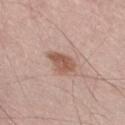Notes:
– follow-up: total-body-photography surveillance lesion; no biopsy
– anatomic site: the right thigh
– illumination: white-light
– imaging modality: ~15 mm crop, total-body skin-cancer survey
– subject: male, aged 53 to 57